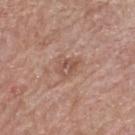This lesion was catalogued during total-body skin photography and was not selected for biopsy.